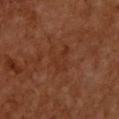| feature | finding |
|---|---|
| biopsy status | no biopsy performed (imaged during a skin exam) |
| acquisition | total-body-photography crop, ~15 mm field of view |
| size | about 4 mm |
| automated metrics | an area of roughly 6.5 mm², a shape eccentricity near 0.7, and a shape-asymmetry score of about 0.5 (0 = symmetric); a normalized border contrast of about 4.5; a nevus-likeness score of about 0/100 and a lesion-detection confidence of about 100/100 |
| illumination | cross-polarized illumination |
| patient | male, aged 58–62 |
| location | the back |